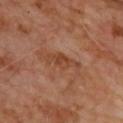Captured during whole-body skin photography for melanoma surveillance; the lesion was not biopsied. This image is a 15 mm lesion crop taken from a total-body photograph. The total-body-photography lesion software estimated an area of roughly 5.5 mm², an eccentricity of roughly 0.95, and two-axis asymmetry of about 0.35. And it measured a lesion color around L≈42 a*≈23 b*≈31 in CIELAB, roughly 7 lightness units darker than nearby skin, and a normalized lesion–skin contrast near 6. A male patient approximately 70 years of age. The tile uses cross-polarized illumination. Located on the upper back. Measured at roughly 4.5 mm in maximum diameter.